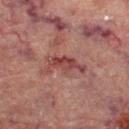Impression:
Captured during whole-body skin photography for melanoma surveillance; the lesion was not biopsied.
Image and clinical context:
The lesion is on the right lower leg. Captured under cross-polarized illumination. The subject is a female approximately 70 years of age. Measured at roughly 5 mm in maximum diameter. Automated tile analysis of the lesion measured a footprint of about 6.5 mm², an eccentricity of roughly 0.95, and a symmetry-axis asymmetry near 0.45. And it measured a lesion color around L≈36 a*≈23 b*≈19 in CIELAB and a normalized border contrast of about 8. The analysis additionally found a border-irregularity index near 7/10, a color-variation rating of about 3/10, and radial color variation of about 1. A lesion tile, about 15 mm wide, cut from a 3D total-body photograph.